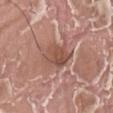{"biopsy_status": "not biopsied; imaged during a skin examination", "image": {"source": "total-body photography crop", "field_of_view_mm": 15}, "automated_metrics": {"area_mm2_approx": 9.0, "eccentricity": 0.7, "shape_asymmetry": 0.2, "cielab_L": 51, "cielab_a": 22, "cielab_b": 27, "vs_skin_darker_L": 10.0, "vs_skin_contrast_norm": 7.0, "nevus_likeness_0_100": 0, "lesion_detection_confidence_0_100": 60}, "lesion_size": {"long_diameter_mm_approx": 4.0}, "patient": {"sex": "male", "age_approx": 40}, "site": "left thigh"}